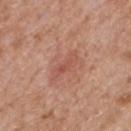<case>
  <biopsy_status>not biopsied; imaged during a skin examination</biopsy_status>
  <lesion_size>
    <long_diameter_mm_approx>4.0</long_diameter_mm_approx>
  </lesion_size>
  <lighting>white-light</lighting>
  <site>mid back</site>
  <image>
    <source>total-body photography crop</source>
    <field_of_view_mm>15</field_of_view_mm>
  </image>
  <patient>
    <sex>male</sex>
    <age_approx>65</age_approx>
  </patient>
</case>